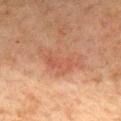Case summary:
– workup: total-body-photography surveillance lesion; no biopsy
– patient: female, in their 60s
– image-analysis metrics: a footprint of about 11 mm² and a shape eccentricity near 0.9; a color-variation rating of about 2/10 and peripheral color asymmetry of about 0.5
– location: the chest
– lesion diameter: ~6 mm (longest diameter)
– lighting: cross-polarized illumination
– acquisition: ~15 mm tile from a whole-body skin photo